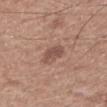Q: Is there a histopathology result?
A: catalogued during a skin exam; not biopsied
Q: What kind of image is this?
A: 15 mm crop, total-body photography
Q: What are the patient's age and sex?
A: male, in their 60s
Q: How large is the lesion?
A: about 3.5 mm
Q: Illumination type?
A: white-light illumination
Q: Where on the body is the lesion?
A: the upper back